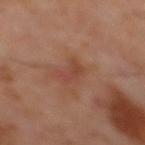No biopsy was performed on this lesion — it was imaged during a full skin examination and was not determined to be concerning. A close-up tile cropped from a whole-body skin photograph, about 15 mm across. This is a cross-polarized tile. The recorded lesion diameter is about 3 mm. The lesion is on the mid back. Automated image analysis of the tile measured a lesion area of about 4 mm² and two-axis asymmetry of about 0.45. And it measured a lesion–skin lightness drop of about 6. It also reported a border-irregularity index near 5/10, a color-variation rating of about 0.5/10, and a peripheral color-asymmetry measure near 0. It also reported a nevus-likeness score of about 0/100. A male subject in their 60s.